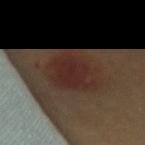Imaged during a routine full-body skin examination; the lesion was not biopsied and no histopathology is available. Automated tile analysis of the lesion measured an area of roughly 18 mm², an outline eccentricity of about 0.6 (0 = round, 1 = elongated), and a shape-asymmetry score of about 0.25 (0 = symmetric). And it measured a nevus-likeness score of about 80/100 and lesion-presence confidence of about 100/100. A close-up tile cropped from a whole-body skin photograph, about 15 mm across. Located on the mid back. A female subject roughly 60 years of age. The lesion's longest dimension is about 5.5 mm.